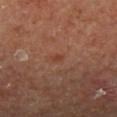Case summary:
* workup · imaged on a skin check; not biopsied
* automated metrics · an area of roughly 1 mm², a shape eccentricity near 0.5, and a symmetry-axis asymmetry near 0.3; border irregularity of about 2 on a 0–10 scale, internal color variation of about 0 on a 0–10 scale, and peripheral color asymmetry of about 0; a classifier nevus-likeness of about 5/100 and a lesion-detection confidence of about 100/100
* patient · male, about 65 years old
* site · the leg
* imaging modality · ~15 mm crop, total-body skin-cancer survey
* lighting · cross-polarized
* lesion size · ~1 mm (longest diameter)